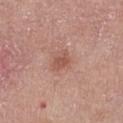Clinical impression: Part of a total-body skin-imaging series; this lesion was reviewed on a skin check and was not flagged for biopsy. Acquisition and patient details: This image is a 15 mm lesion crop taken from a total-body photograph. The lesion-visualizer software estimated an area of roughly 4.5 mm², an eccentricity of roughly 0.65, and two-axis asymmetry of about 0.25. The analysis additionally found about 8 CIELAB-L* units darker than the surrounding skin and a normalized border contrast of about 6. The lesion is located on the right thigh. A female subject, in their mid- to late 60s. Measured at roughly 2.5 mm in maximum diameter. The tile uses white-light illumination.